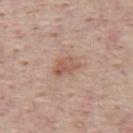- biopsy status — total-body-photography surveillance lesion; no biopsy
- subject — male, aged around 45
- image source — 15 mm crop, total-body photography
- location — the mid back
- automated lesion analysis — an eccentricity of roughly 0.8; an average lesion color of about L≈56 a*≈21 b*≈28 (CIELAB), about 9 CIELAB-L* units darker than the surrounding skin, and a normalized lesion–skin contrast near 6.5; a within-lesion color-variation index near 3/10 and peripheral color asymmetry of about 1; a lesion-detection confidence of about 100/100
- lesion size — ≈3.5 mm
- illumination — white-light illumination